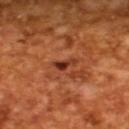Assessment:
Part of a total-body skin-imaging series; this lesion was reviewed on a skin check and was not flagged for biopsy.
Context:
The patient is a male aged 63 to 67. Longest diameter approximately 2.5 mm. A lesion tile, about 15 mm wide, cut from a 3D total-body photograph. Captured under cross-polarized illumination.